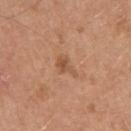body site: the left upper arm | tile lighting: white-light | TBP lesion metrics: an average lesion color of about L≈53 a*≈22 b*≈34 (CIELAB), a lesion–skin lightness drop of about 8, and a lesion-to-skin contrast of about 6 (normalized; higher = more distinct); a nevus-likeness score of about 20/100 and a lesion-detection confidence of about 100/100 | subject: male, aged around 75 | image source: 15 mm crop, total-body photography | lesion size: ≈3 mm.